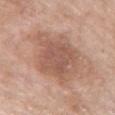Q: Is there a histopathology result?
A: catalogued during a skin exam; not biopsied
Q: What are the patient's age and sex?
A: female, approximately 75 years of age
Q: Lesion location?
A: the chest
Q: How was the tile lit?
A: white-light
Q: How was this image acquired?
A: ~15 mm crop, total-body skin-cancer survey
Q: Lesion size?
A: ~7.5 mm (longest diameter)